A male subject, roughly 60 years of age. The tile uses white-light illumination. Cropped from a whole-body photographic skin survey; the tile spans about 15 mm. Automated tile analysis of the lesion measured a footprint of about 7 mm², an outline eccentricity of about 0.9 (0 = round, 1 = elongated), and a symmetry-axis asymmetry near 0.35. Longest diameter approximately 4.5 mm. On the head or neck:
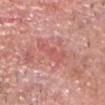| key | value |
|---|---|
| histopathology | a squamous cell carcinoma in situ — a malignant skin lesion |